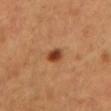<lesion>
<biopsy_status>not biopsied; imaged during a skin examination</biopsy_status>
<site>front of the torso</site>
<image>
  <source>total-body photography crop</source>
  <field_of_view_mm>15</field_of_view_mm>
</image>
<lighting>cross-polarized</lighting>
<patient>
  <sex>female</sex>
  <age_approx>55</age_approx>
</patient>
<lesion_size>
  <long_diameter_mm_approx>2.5</long_diameter_mm_approx>
</lesion_size>
</lesion>